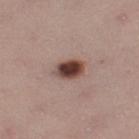Recorded during total-body skin imaging; not selected for excision or biopsy. The patient is a female aged around 25. Captured under white-light illumination. A lesion tile, about 15 mm wide, cut from a 3D total-body photograph. The lesion is located on the leg. Automated image analysis of the tile measured a mean CIELAB color near L≈43 a*≈19 b*≈22, roughly 18 lightness units darker than nearby skin, and a normalized lesion–skin contrast near 12.5. It also reported an automated nevus-likeness rating near 100 out of 100 and lesion-presence confidence of about 100/100. Measured at roughly 4 mm in maximum diameter.